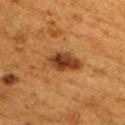notes: total-body-photography surveillance lesion; no biopsy
location: the right upper arm
subject: female, in their 50s
image source: total-body-photography crop, ~15 mm field of view
diameter: about 5 mm
automated lesion analysis: an area of roughly 9.5 mm² and an eccentricity of roughly 0.85; a border-irregularity rating of about 3/10, a within-lesion color-variation index near 6.5/10, and radial color variation of about 2; a classifier nevus-likeness of about 85/100 and a lesion-detection confidence of about 100/100
illumination: cross-polarized